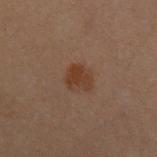Imaged during a routine full-body skin examination; the lesion was not biopsied and no histopathology is available. Located on the left upper arm. The tile uses cross-polarized illumination. Automated tile analysis of the lesion measured a border-irregularity rating of about 2.5/10 and a color-variation rating of about 3/10. It also reported an automated nevus-likeness rating near 80 out of 100 and a detector confidence of about 100 out of 100 that the crop contains a lesion. A female patient, about 30 years old. A 15 mm close-up extracted from a 3D total-body photography capture. The lesion's longest dimension is about 3 mm.